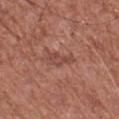<record>
<image>
  <source>total-body photography crop</source>
  <field_of_view_mm>15</field_of_view_mm>
</image>
<lighting>white-light</lighting>
<site>chest</site>
<automated_metrics>
  <cielab_L>46</cielab_L>
  <cielab_a>24</cielab_a>
  <cielab_b>26</cielab_b>
  <vs_skin_contrast_norm>6.0</vs_skin_contrast_norm>
  <border_irregularity_0_10>4.0</border_irregularity_0_10>
  <color_variation_0_10>1.5</color_variation_0_10>
  <peripheral_color_asymmetry>0.5</peripheral_color_asymmetry>
</automated_metrics>
<patient>
  <sex>male</sex>
  <age_approx>75</age_approx>
</patient>
</record>